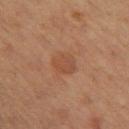Recorded during total-body skin imaging; not selected for excision or biopsy. A region of skin cropped from a whole-body photographic capture, roughly 15 mm wide. An algorithmic analysis of the crop reported a footprint of about 5.5 mm² and a shape eccentricity near 0.45. The analysis additionally found a within-lesion color-variation index near 2.5/10. And it measured an automated nevus-likeness rating near 0 out of 100. This is a cross-polarized tile. The lesion is located on the left thigh. The patient is a female aged approximately 40.